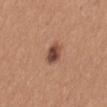Impression:
Part of a total-body skin-imaging series; this lesion was reviewed on a skin check and was not flagged for biopsy.